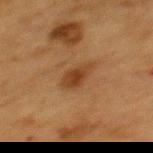<case>
  <biopsy_status>not biopsied; imaged during a skin examination</biopsy_status>
  <patient>
    <sex>female</sex>
    <age_approx>55</age_approx>
  </patient>
  <automated_metrics>
    <eccentricity>0.75</eccentricity>
    <shape_asymmetry>0.2</shape_asymmetry>
    <cielab_L>33</cielab_L>
    <cielab_a>20</cielab_a>
    <cielab_b>31</cielab_b>
    <vs_skin_contrast_norm>8.0</vs_skin_contrast_norm>
  </automated_metrics>
  <image>
    <source>total-body photography crop</source>
    <field_of_view_mm>15</field_of_view_mm>
  </image>
  <lighting>cross-polarized</lighting>
  <lesion_size>
    <long_diameter_mm_approx>3.0</long_diameter_mm_approx>
  </lesion_size>
  <site>upper back</site>
</case>